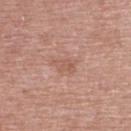{
  "biopsy_status": "not biopsied; imaged during a skin examination",
  "lesion_size": {
    "long_diameter_mm_approx": 3.0
  },
  "patient": {
    "sex": "female",
    "age_approx": 60
  },
  "image": {
    "source": "total-body photography crop",
    "field_of_view_mm": 15
  },
  "site": "upper back",
  "lighting": "white-light"
}